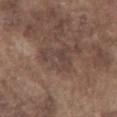Recorded during total-body skin imaging; not selected for excision or biopsy. A male subject in their mid-70s. Cropped from a whole-body photographic skin survey; the tile spans about 15 mm. The lesion is on the chest.